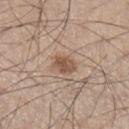Q: Is there a histopathology result?
A: total-body-photography surveillance lesion; no biopsy
Q: What did automated image analysis measure?
A: a mean CIELAB color near L≈54 a*≈17 b*≈28, a lesion–skin lightness drop of about 10, and a normalized lesion–skin contrast near 7.5; a within-lesion color-variation index near 3/10 and a peripheral color-asymmetry measure near 1
Q: Illumination type?
A: white-light
Q: Who is the patient?
A: male, about 45 years old
Q: How was this image acquired?
A: ~15 mm crop, total-body skin-cancer survey
Q: What is the anatomic site?
A: the left lower leg